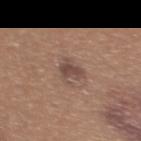Recorded during total-body skin imaging; not selected for excision or biopsy.
A close-up tile cropped from a whole-body skin photograph, about 15 mm across.
Measured at roughly 3 mm in maximum diameter.
The lesion is on the upper back.
The subject is a female aged 38–42.
Captured under white-light illumination.
Automated tile analysis of the lesion measured an area of roughly 4.5 mm² and an outline eccentricity of about 0.65 (0 = round, 1 = elongated). It also reported an average lesion color of about L≈46 a*≈17 b*≈23 (CIELAB) and a lesion-to-skin contrast of about 7.5 (normalized; higher = more distinct). The software also gave a border-irregularity index near 3.5/10, a within-lesion color-variation index near 2.5/10, and a peripheral color-asymmetry measure near 1. And it measured a classifier nevus-likeness of about 35/100 and lesion-presence confidence of about 100/100.